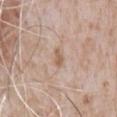Case summary:
• illumination — white-light
• automated lesion analysis — a lesion area of about 2.5 mm², an outline eccentricity of about 0.9 (0 = round, 1 = elongated), and two-axis asymmetry of about 0.3; a lesion color around L≈59 a*≈17 b*≈29 in CIELAB, roughly 9 lightness units darker than nearby skin, and a normalized lesion–skin contrast near 6.5; a color-variation rating of about 0/10 and peripheral color asymmetry of about 0; an automated nevus-likeness rating near 0 out of 100 and lesion-presence confidence of about 100/100
• anatomic site — the chest
• patient — male, aged approximately 65
• lesion diameter — ≈2.5 mm
• image source — ~15 mm tile from a whole-body skin photo